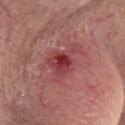Notes:
– lighting · white-light illumination
– lesion diameter · about 5 mm
– anatomic site · the head or neck
– imaging modality · total-body-photography crop, ~15 mm field of view
– subject · female, approximately 65 years of age